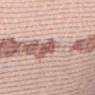The lesion was photographed on a routine skin check and not biopsied; there is no pathology result.
A female patient aged 38–42.
Located on the abdomen.
This image is a 15 mm lesion crop taken from a total-body photograph.